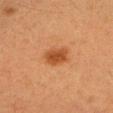biopsy status: catalogued during a skin exam; not biopsied
lesion diameter: ≈3 mm
patient: female, about 40 years old
image-analysis metrics: a footprint of about 6 mm², an eccentricity of roughly 0.65, and two-axis asymmetry of about 0.2; a mean CIELAB color near L≈42 a*≈24 b*≈36, about 10 CIELAB-L* units darker than the surrounding skin, and a lesion-to-skin contrast of about 8.5 (normalized; higher = more distinct); a color-variation rating of about 2/10 and peripheral color asymmetry of about 0.5; a lesion-detection confidence of about 100/100
image: ~15 mm crop, total-body skin-cancer survey
anatomic site: the head or neck